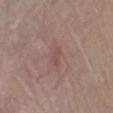{
  "biopsy_status": "not biopsied; imaged during a skin examination",
  "lighting": "white-light",
  "automated_metrics": {
    "cielab_L": 49,
    "cielab_a": 21,
    "cielab_b": 21,
    "vs_skin_darker_L": 6.0,
    "vs_skin_contrast_norm": 4.5,
    "border_irregularity_0_10": 3.5
  },
  "site": "right lower leg",
  "image": {
    "source": "total-body photography crop",
    "field_of_view_mm": 15
  },
  "patient": {
    "sex": "male",
    "age_approx": 55
  },
  "lesion_size": {
    "long_diameter_mm_approx": 2.5
  }
}